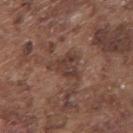{"biopsy_status": "not biopsied; imaged during a skin examination"}